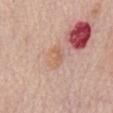Assessment:
This lesion was catalogued during total-body skin photography and was not selected for biopsy.
Clinical summary:
The patient is a female aged 63–67. About 3 mm across. A 15 mm close-up extracted from a 3D total-body photography capture. Imaged with white-light lighting. The lesion is on the chest.